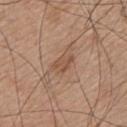This lesion was catalogued during total-body skin photography and was not selected for biopsy. Imaged with white-light lighting. A male subject, about 65 years old. Cropped from a whole-body photographic skin survey; the tile spans about 15 mm. From the mid back.